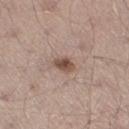notes: catalogued during a skin exam; not biopsied
anatomic site: the right thigh
image source: 15 mm crop, total-body photography
subject: male, roughly 35 years of age